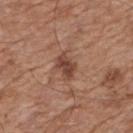Case summary:
– workup: imaged on a skin check; not biopsied
– anatomic site: the upper back
– TBP lesion metrics: a mean CIELAB color near L≈44 a*≈21 b*≈27, a lesion–skin lightness drop of about 11, and a normalized border contrast of about 8.5; border irregularity of about 2.5 on a 0–10 scale and peripheral color asymmetry of about 1; a nevus-likeness score of about 20/100 and a lesion-detection confidence of about 100/100
– image: ~15 mm tile from a whole-body skin photo
– lighting: white-light illumination
– lesion diameter: about 3 mm
– subject: male, roughly 65 years of age